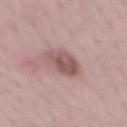{
  "biopsy_status": "not biopsied; imaged during a skin examination",
  "image": {
    "source": "total-body photography crop",
    "field_of_view_mm": 15
  },
  "patient": {
    "sex": "male",
    "age_approx": 50
  },
  "lesion_size": {
    "long_diameter_mm_approx": 4.5
  },
  "site": "back",
  "automated_metrics": {
    "area_mm2_approx": 9.0,
    "shape_asymmetry": 0.2,
    "color_variation_0_10": 4.5,
    "peripheral_color_asymmetry": 2.0,
    "nevus_likeness_0_100": 10,
    "lesion_detection_confidence_0_100": 100
  },
  "lighting": "white-light"
}